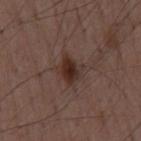The lesion was tiled from a total-body skin photograph and was not biopsied.
The patient is a male aged 48–52.
This is a white-light tile.
Located on the back.
A 15 mm close-up extracted from a 3D total-body photography capture.